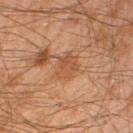The lesion was photographed on a routine skin check and not biopsied; there is no pathology result. The lesion-visualizer software estimated a within-lesion color-variation index near 2.5/10. It also reported a nevus-likeness score of about 0/100 and lesion-presence confidence of about 100/100. On the right lower leg. A region of skin cropped from a whole-body photographic capture, roughly 15 mm wide. The subject is a male in their mid- to late 40s. The tile uses cross-polarized illumination.